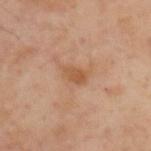Recorded during total-body skin imaging; not selected for excision or biopsy. Imaged with cross-polarized lighting. The total-body-photography lesion software estimated a lesion area of about 4 mm², an eccentricity of roughly 0.8, and a shape-asymmetry score of about 0.25 (0 = symmetric). The software also gave an average lesion color of about L≈54 a*≈21 b*≈36 (CIELAB), a lesion–skin lightness drop of about 8, and a normalized lesion–skin contrast near 7. And it measured a border-irregularity index near 2.5/10, internal color variation of about 2 on a 0–10 scale, and radial color variation of about 0.5. It also reported an automated nevus-likeness rating near 0 out of 100 and lesion-presence confidence of about 100/100. The lesion's longest dimension is about 3 mm. A male subject aged around 55. A lesion tile, about 15 mm wide, cut from a 3D total-body photograph. The lesion is located on the upper back.